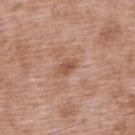Imaged during a routine full-body skin examination; the lesion was not biopsied and no histopathology is available.
This is a white-light tile.
The lesion's longest dimension is about 2.5 mm.
From the upper back.
A 15 mm close-up extracted from a 3D total-body photography capture.
Automated tile analysis of the lesion measured an automated nevus-likeness rating near 0 out of 100 and lesion-presence confidence of about 100/100.
The patient is a male aged approximately 50.